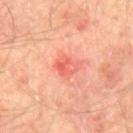Case summary:
* follow-up — catalogued during a skin exam; not biopsied
* patient — male, roughly 65 years of age
* body site — the mid back
* image — total-body-photography crop, ~15 mm field of view
* tile lighting — cross-polarized illumination